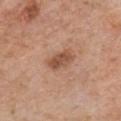A male subject aged 58–62. A roughly 15 mm field-of-view crop from a total-body skin photograph. Captured under white-light illumination. Measured at roughly 3.5 mm in maximum diameter. From the front of the torso.